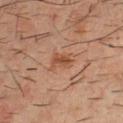Recorded during total-body skin imaging; not selected for excision or biopsy. The lesion is located on the chest. A male patient, in their mid- to late 30s. A close-up tile cropped from a whole-body skin photograph, about 15 mm across.